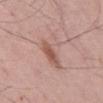Recorded during total-body skin imaging; not selected for excision or biopsy. An algorithmic analysis of the crop reported a lesion area of about 11 mm², an outline eccentricity of about 0.7 (0 = round, 1 = elongated), and two-axis asymmetry of about 0.45. The software also gave a mean CIELAB color near L≈58 a*≈20 b*≈24, about 8 CIELAB-L* units darker than the surrounding skin, and a normalized lesion–skin contrast near 5.5. The analysis additionally found border irregularity of about 5.5 on a 0–10 scale, a color-variation rating of about 6.5/10, and a peripheral color-asymmetry measure near 2. It also reported lesion-presence confidence of about 100/100. A male patient, about 55 years old. The lesion is on the mid back. The tile uses white-light illumination. Approximately 4.5 mm at its widest. A 15 mm close-up tile from a total-body photography series done for melanoma screening.